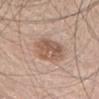workup: catalogued during a skin exam; not biopsied
patient: female, in their 30s
size: ~4.5 mm (longest diameter)
tile lighting: white-light illumination
body site: the head or neck
image: total-body-photography crop, ~15 mm field of view